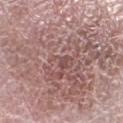Q: Was a biopsy performed?
A: total-body-photography surveillance lesion; no biopsy
Q: Where on the body is the lesion?
A: the left lower leg
Q: What is the imaging modality?
A: ~15 mm tile from a whole-body skin photo
Q: Patient demographics?
A: female, in their 60s